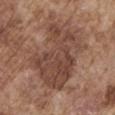Captured during whole-body skin photography for melanoma surveillance; the lesion was not biopsied.